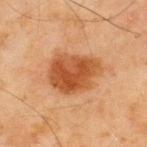Acquisition and patient details: A male subject, aged 43–47. Imaged with cross-polarized lighting. From the upper back. Automated image analysis of the tile measured a footprint of about 19 mm² and an outline eccentricity of about 0.65 (0 = round, 1 = elongated). It also reported an average lesion color of about L≈43 a*≈23 b*≈34 (CIELAB) and a lesion–skin lightness drop of about 11. The software also gave an automated nevus-likeness rating near 100 out of 100 and a lesion-detection confidence of about 100/100. A lesion tile, about 15 mm wide, cut from a 3D total-body photograph. Approximately 5.5 mm at its widest. Conclusion: Histopathology of the biopsied lesion showed a melanoma in situ.